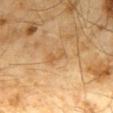Notes:
* biopsy status — imaged on a skin check; not biopsied
* illumination — cross-polarized illumination
* image — ~15 mm crop, total-body skin-cancer survey
* subject — male, roughly 65 years of age
* body site — the back
* lesion size — ≈2.5 mm
* automated metrics — a mean CIELAB color near L≈55 a*≈18 b*≈39, a lesion–skin lightness drop of about 6, and a normalized lesion–skin contrast near 5; a border-irregularity rating of about 4/10, a color-variation rating of about 3/10, and peripheral color asymmetry of about 1; a classifier nevus-likeness of about 0/100 and a detector confidence of about 100 out of 100 that the crop contains a lesion